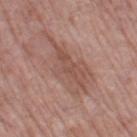{
  "biopsy_status": "not biopsied; imaged during a skin examination",
  "patient": {
    "sex": "female",
    "age_approx": 60
  },
  "lesion_size": {
    "long_diameter_mm_approx": 8.5
  },
  "image": {
    "source": "total-body photography crop",
    "field_of_view_mm": 15
  },
  "lighting": "white-light",
  "automated_metrics": {
    "border_irregularity_0_10": 7.0,
    "color_variation_0_10": 4.0,
    "peripheral_color_asymmetry": 1.5,
    "nevus_likeness_0_100": 0,
    "lesion_detection_confidence_0_100": 95
  },
  "site": "left thigh"
}